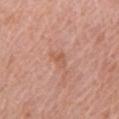notes: total-body-photography surveillance lesion; no biopsy | tile lighting: white-light | automated metrics: a shape eccentricity near 0.8 and a shape-asymmetry score of about 0.45 (0 = symmetric); a mean CIELAB color near L≈57 a*≈23 b*≈32 and about 7 CIELAB-L* units darker than the surrounding skin; a border-irregularity index near 4.5/10 and peripheral color asymmetry of about 0.5; a classifier nevus-likeness of about 0/100 and a detector confidence of about 100 out of 100 that the crop contains a lesion | image source: total-body-photography crop, ~15 mm field of view | subject: female, aged around 60 | body site: the left upper arm | diameter: ≈2.5 mm.